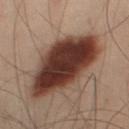Clinical impression:
The lesion was tiled from a total-body skin photograph and was not biopsied.
Background:
A roughly 15 mm field-of-view crop from a total-body skin photograph. The subject is a male aged 48–52. Longest diameter approximately 10 mm. The tile uses cross-polarized illumination. Located on the left leg.